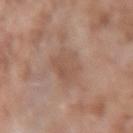Recorded during total-body skin imaging; not selected for excision or biopsy. Approximately 4.5 mm at its widest. On the right forearm. A female patient about 75 years old. Automated tile analysis of the lesion measured an area of roughly 10 mm². It also reported an average lesion color of about L≈53 a*≈18 b*≈27 (CIELAB), about 7 CIELAB-L* units darker than the surrounding skin, and a normalized lesion–skin contrast near 5. This image is a 15 mm lesion crop taken from a total-body photograph.